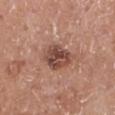<tbp_lesion>
<patient>
  <sex>male</sex>
  <age_approx>55</age_approx>
</patient>
<image>
  <source>total-body photography crop</source>
  <field_of_view_mm>15</field_of_view_mm>
</image>
<site>leg</site>
<lesion_size>
  <long_diameter_mm_approx>4.0</long_diameter_mm_approx>
</lesion_size>
</tbp_lesion>